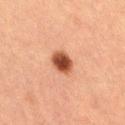{"site": "right thigh", "lighting": "cross-polarized", "lesion_size": {"long_diameter_mm_approx": 3.0}, "image": {"source": "total-body photography crop", "field_of_view_mm": 15}, "patient": {"sex": "female", "age_approx": 40}, "automated_metrics": {"eccentricity": 0.55, "shape_asymmetry": 0.2, "border_irregularity_0_10": 1.5, "color_variation_0_10": 4.5, "peripheral_color_asymmetry": 1.5, "lesion_detection_confidence_0_100": 100}}